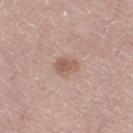No biopsy was performed on this lesion — it was imaged during a full skin examination and was not determined to be concerning. The lesion is on the right thigh. Captured under white-light illumination. A lesion tile, about 15 mm wide, cut from a 3D total-body photograph. A female subject aged 63 to 67.